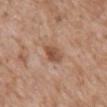follow-up: no biopsy performed (imaged during a skin exam)
tile lighting: white-light illumination
anatomic site: the front of the torso
image source: 15 mm crop, total-body photography
automated lesion analysis: a footprint of about 5 mm², an outline eccentricity of about 0.7 (0 = round, 1 = elongated), and a shape-asymmetry score of about 0.2 (0 = symmetric); a mean CIELAB color near L≈51 a*≈21 b*≈30 and about 11 CIELAB-L* units darker than the surrounding skin; an automated nevus-likeness rating near 45 out of 100
subject: male, approximately 60 years of age
size: ~3 mm (longest diameter)